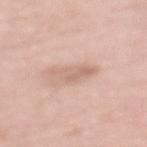Q: Illumination type?
A: white-light
Q: Where on the body is the lesion?
A: the mid back
Q: How large is the lesion?
A: ≈4 mm
Q: Who is the patient?
A: female, aged 68 to 72
Q: Automated lesion metrics?
A: a lesion–skin lightness drop of about 9 and a normalized lesion–skin contrast near 5.5
Q: How was this image acquired?
A: ~15 mm crop, total-body skin-cancer survey